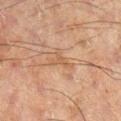| field | value |
|---|---|
| follow-up | total-body-photography surveillance lesion; no biopsy |
| diameter | ≈3.5 mm |
| acquisition | ~15 mm tile from a whole-body skin photo |
| subject | male, aged around 60 |
| tile lighting | cross-polarized |
| anatomic site | the left leg |